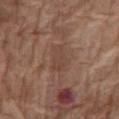Case summary:
– notes · catalogued during a skin exam; not biopsied
– diameter · about 3.5 mm
– anatomic site · the abdomen
– patient · female, roughly 75 years of age
– imaging modality · ~15 mm tile from a whole-body skin photo
– lighting · white-light
– automated metrics · a footprint of about 5.5 mm² and a shape eccentricity near 0.8; a color-variation rating of about 1.5/10 and peripheral color asymmetry of about 0.5; a classifier nevus-likeness of about 0/100 and a detector confidence of about 100 out of 100 that the crop contains a lesion